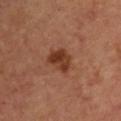This lesion was catalogued during total-body skin photography and was not selected for biopsy. Longest diameter approximately 3 mm. Cropped from a total-body skin-imaging series; the visible field is about 15 mm. Imaged with cross-polarized lighting. Located on the chest. A female subject about 45 years old.